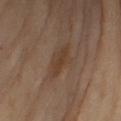Impression: Imaged during a routine full-body skin examination; the lesion was not biopsied and no histopathology is available. Clinical summary: A female patient, aged 53–57. About 4 mm across. A roughly 15 mm field-of-view crop from a total-body skin photograph. The lesion is located on the left upper arm. This is a cross-polarized tile.